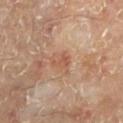Captured during whole-body skin photography for melanoma surveillance; the lesion was not biopsied.
Imaged with cross-polarized lighting.
Approximately 2.5 mm at its widest.
Cropped from a total-body skin-imaging series; the visible field is about 15 mm.
The lesion is on the left lower leg.
A subject about 55 years old.